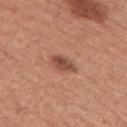workup = total-body-photography surveillance lesion; no biopsy | tile lighting = white-light | diameter = ≈3.5 mm | imaging modality = ~15 mm crop, total-body skin-cancer survey | patient = female, about 50 years old | body site = the right upper arm.